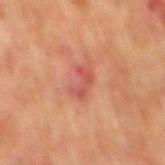Clinical impression: Part of a total-body skin-imaging series; this lesion was reviewed on a skin check and was not flagged for biopsy. Clinical summary: Located on the mid back. A region of skin cropped from a whole-body photographic capture, roughly 15 mm wide. Approximately 3 mm at its widest. The subject is a male aged 58 to 62. The tile uses cross-polarized illumination.